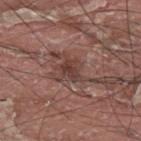Clinical impression:
Recorded during total-body skin imaging; not selected for excision or biopsy.
Acquisition and patient details:
Approximately 2.5 mm at its widest. Cropped from a whole-body photographic skin survey; the tile spans about 15 mm. A male patient roughly 40 years of age. The lesion is on the upper back.